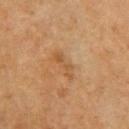No biopsy was performed on this lesion — it was imaged during a full skin examination and was not determined to be concerning.
A female subject aged 53 to 57.
On the arm.
Cropped from a whole-body photographic skin survey; the tile spans about 15 mm.